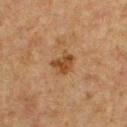Part of a total-body skin-imaging series; this lesion was reviewed on a skin check and was not flagged for biopsy.
A lesion tile, about 15 mm wide, cut from a 3D total-body photograph.
A male subject, aged 73 to 77.
The lesion is on the chest.